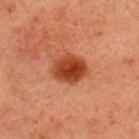{"biopsy_status": "not biopsied; imaged during a skin examination", "patient": {"sex": "male", "age_approx": 60}, "automated_metrics": {"cielab_L": 33, "cielab_a": 26, "cielab_b": 31, "vs_skin_darker_L": 12.0, "vs_skin_contrast_norm": 11.0, "border_irregularity_0_10": 1.5, "peripheral_color_asymmetry": 1.5, "nevus_likeness_0_100": 100}, "lesion_size": {"long_diameter_mm_approx": 4.0}, "site": "upper back", "image": {"source": "total-body photography crop", "field_of_view_mm": 15}, "lighting": "cross-polarized"}